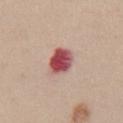No biopsy was performed on this lesion — it was imaged during a full skin examination and was not determined to be concerning. Approximately 3.5 mm at its widest. A female subject, in their 40s. A roughly 15 mm field-of-view crop from a total-body skin photograph. From the abdomen.